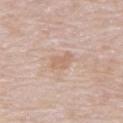Q: Lesion location?
A: the abdomen
Q: Who is the patient?
A: male, approximately 80 years of age
Q: Illumination type?
A: white-light
Q: Lesion size?
A: ~3 mm (longest diameter)
Q: How was this image acquired?
A: ~15 mm crop, total-body skin-cancer survey